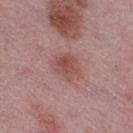Q: Is there a histopathology result?
A: imaged on a skin check; not biopsied
Q: What kind of image is this?
A: 15 mm crop, total-body photography
Q: Patient demographics?
A: female, in their mid- to late 40s
Q: Lesion location?
A: the right thigh